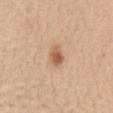notes = total-body-photography surveillance lesion; no biopsy
subject = female, aged approximately 65
image source = total-body-photography crop, ~15 mm field of view
lesion size = ≈2.5 mm
TBP lesion metrics = an eccentricity of roughly 0.65 and two-axis asymmetry of about 0.25; an average lesion color of about L≈60 a*≈20 b*≈34 (CIELAB), roughly 11 lightness units darker than nearby skin, and a normalized border contrast of about 7.5; a nevus-likeness score of about 95/100 and a detector confidence of about 100 out of 100 that the crop contains a lesion
site = the abdomen